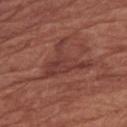Impression: No biopsy was performed on this lesion — it was imaged during a full skin examination and was not determined to be concerning. Context: A roughly 15 mm field-of-view crop from a total-body skin photograph. The lesion is on the right forearm. About 6 mm across. A female patient, aged 73 to 77.